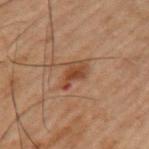This lesion was catalogued during total-body skin photography and was not selected for biopsy.
This image is a 15 mm lesion crop taken from a total-body photograph.
Located on the left upper arm.
A male patient, in their 60s.